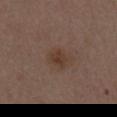Clinical impression:
The lesion was photographed on a routine skin check and not biopsied; there is no pathology result.
Context:
Cropped from a whole-body photographic skin survey; the tile spans about 15 mm. The recorded lesion diameter is about 2.5 mm. On the abdomen. The tile uses white-light illumination. A male subject aged around 50.